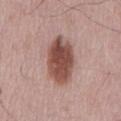Case summary:
- notes — no biopsy performed (imaged during a skin exam)
- body site — the mid back
- subject — male, approximately 65 years of age
- diameter — ≈6 mm
- tile lighting — white-light
- TBP lesion metrics — border irregularity of about 1.5 on a 0–10 scale; an automated nevus-likeness rating near 100 out of 100 and a lesion-detection confidence of about 100/100
- acquisition — ~15 mm crop, total-body skin-cancer survey